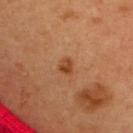biopsy status = catalogued during a skin exam; not biopsied
patient = female, aged 33 to 37
site = the upper back
imaging modality = 15 mm crop, total-body photography
TBP lesion metrics = a lesion color around L≈44 a*≈26 b*≈38 in CIELAB and about 10 CIELAB-L* units darker than the surrounding skin; lesion-presence confidence of about 100/100
lighting = cross-polarized illumination
size = ~2 mm (longest diameter)